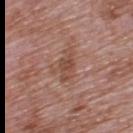Clinical impression: The lesion was tiled from a total-body skin photograph and was not biopsied. Image and clinical context: Longest diameter approximately 4 mm. The total-body-photography lesion software estimated a footprint of about 6.5 mm² and a symmetry-axis asymmetry near 0.35. The analysis additionally found a classifier nevus-likeness of about 0/100 and a lesion-detection confidence of about 100/100. A 15 mm close-up extracted from a 3D total-body photography capture. A male subject about 70 years old. On the back. Captured under white-light illumination.